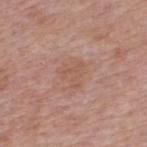A male subject, about 55 years old. The lesion is on the upper back. Longest diameter approximately 3.5 mm. A 15 mm close-up tile from a total-body photography series done for melanoma screening. The tile uses white-light illumination.